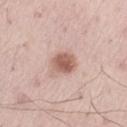Impression:
The lesion was tiled from a total-body skin photograph and was not biopsied.
Context:
The patient is a male in their mid- to late 50s. The lesion is on the left thigh. Cropped from a whole-body photographic skin survey; the tile spans about 15 mm.